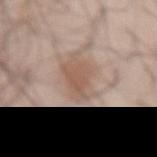follow-up — imaged on a skin check; not biopsied | lesion size — ≈5 mm | automated lesion analysis — a lesion–skin lightness drop of about 9 and a lesion-to-skin contrast of about 6.5 (normalized; higher = more distinct); a border-irregularity rating of about 3.5/10, a within-lesion color-variation index near 3/10, and radial color variation of about 1; a nevus-likeness score of about 75/100 and a lesion-detection confidence of about 100/100 | body site — the abdomen | patient — male, aged approximately 55 | image — ~15 mm tile from a whole-body skin photo | illumination — white-light.